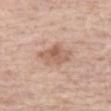Q: Was this lesion biopsied?
A: no biopsy performed (imaged during a skin exam)
Q: Lesion size?
A: about 4.5 mm
Q: Who is the patient?
A: male, aged 78 to 82
Q: How was the tile lit?
A: white-light
Q: Automated lesion metrics?
A: a shape eccentricity near 0.8 and a shape-asymmetry score of about 0.25 (0 = symmetric); an average lesion color of about L≈60 a*≈20 b*≈29 (CIELAB), about 10 CIELAB-L* units darker than the surrounding skin, and a normalized border contrast of about 7; an automated nevus-likeness rating near 0 out of 100 and a detector confidence of about 100 out of 100 that the crop contains a lesion
Q: What kind of image is this?
A: 15 mm crop, total-body photography
Q: Lesion location?
A: the left thigh